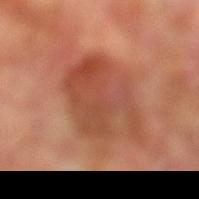Part of a total-body skin-imaging series; this lesion was reviewed on a skin check and was not flagged for biopsy.